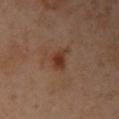Captured during whole-body skin photography for melanoma surveillance; the lesion was not biopsied. A female subject aged approximately 40. The total-body-photography lesion software estimated a lesion area of about 5 mm² and a shape eccentricity near 0.7. The software also gave a border-irregularity rating of about 4/10, a color-variation rating of about 3/10, and a peripheral color-asymmetry measure near 1. Captured under cross-polarized illumination. The lesion is on the left arm. A roughly 15 mm field-of-view crop from a total-body skin photograph. Approximately 3 mm at its widest.